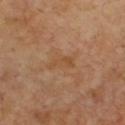notes: total-body-photography surveillance lesion; no biopsy | diameter: about 3.5 mm | TBP lesion metrics: a shape eccentricity near 0.9 and a shape-asymmetry score of about 0.35 (0 = symmetric) | patient: male, aged around 65 | image: total-body-photography crop, ~15 mm field of view.